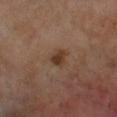Captured during whole-body skin photography for melanoma surveillance; the lesion was not biopsied. Imaged with cross-polarized lighting. On the right lower leg. Cropped from a total-body skin-imaging series; the visible field is about 15 mm. Measured at roughly 2 mm in maximum diameter. The patient is a male aged 68 to 72. The lesion-visualizer software estimated a footprint of about 3.5 mm², an eccentricity of roughly 0.55, and a symmetry-axis asymmetry near 0.35. The analysis additionally found roughly 9 lightness units darker than nearby skin and a lesion-to-skin contrast of about 8.5 (normalized; higher = more distinct). The analysis additionally found a border-irregularity rating of about 3/10 and a within-lesion color-variation index near 2/10. It also reported a nevus-likeness score of about 80/100.